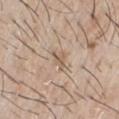workup=total-body-photography surveillance lesion; no biopsy
image=~15 mm tile from a whole-body skin photo
subject=male, aged approximately 65
site=the chest
automated lesion analysis=an area of roughly 2.5 mm² and two-axis asymmetry of about 0.45; a mean CIELAB color near L≈58 a*≈15 b*≈28 and a lesion–skin lightness drop of about 9; a border-irregularity rating of about 5/10 and a peripheral color-asymmetry measure near 0.5
size=~2.5 mm (longest diameter)